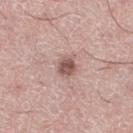{"biopsy_status": "not biopsied; imaged during a skin examination", "patient": {"sex": "male", "age_approx": 50}, "lesion_size": {"long_diameter_mm_approx": 3.0}, "site": "left thigh", "image": {"source": "total-body photography crop", "field_of_view_mm": 15}}